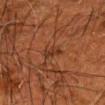Part of a total-body skin-imaging series; this lesion was reviewed on a skin check and was not flagged for biopsy. A roughly 15 mm field-of-view crop from a total-body skin photograph. Located on the head or neck. A male subject, approximately 60 years of age.